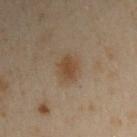Recorded during total-body skin imaging; not selected for excision or biopsy. A lesion tile, about 15 mm wide, cut from a 3D total-body photograph. A male patient aged 48–52. On the left arm. Longest diameter approximately 3.5 mm. Captured under cross-polarized illumination.